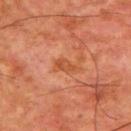notes: catalogued during a skin exam; not biopsied | patient: male, roughly 65 years of age | body site: the upper back | image: 15 mm crop, total-body photography.